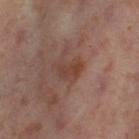Impression: The lesion was tiled from a total-body skin photograph and was not biopsied. Context: A female patient, about 55 years old. Cropped from a total-body skin-imaging series; the visible field is about 15 mm. Captured under cross-polarized illumination. The lesion is on the right thigh. About 3.5 mm across.